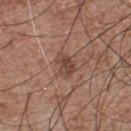biopsy status: catalogued during a skin exam; not biopsied.